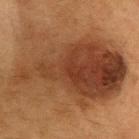Clinical summary:
A lesion tile, about 15 mm wide, cut from a 3D total-body photograph. A male patient aged 53 to 57. On the head or neck.
Conclusion:
On biopsy, histopathology showed a compound melanocytic nevus.